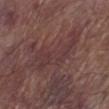Q: Was this lesion biopsied?
A: no biopsy performed (imaged during a skin exam)
Q: Illumination type?
A: white-light
Q: Lesion location?
A: the left lower leg
Q: How was this image acquired?
A: ~15 mm tile from a whole-body skin photo
Q: What is the lesion's diameter?
A: about 7.5 mm
Q: Patient demographics?
A: male, aged approximately 80